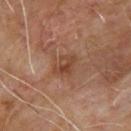Assessment: The lesion was tiled from a total-body skin photograph and was not biopsied. Image and clinical context: Captured under cross-polarized illumination. A roughly 15 mm field-of-view crop from a total-body skin photograph. From the back. A male patient, aged 63 to 67.